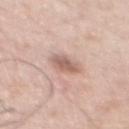biopsy status = imaged on a skin check; not biopsied
subject = male, aged 58 to 62
body site = the chest
image source = 15 mm crop, total-body photography
automated metrics = an average lesion color of about L≈61 a*≈19 b*≈25 (CIELAB), a lesion–skin lightness drop of about 12, and a normalized border contrast of about 7.5; a border-irregularity index near 2.5/10, internal color variation of about 3.5 on a 0–10 scale, and peripheral color asymmetry of about 1.5
lighting = white-light